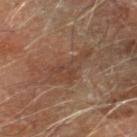Impression:
The lesion was photographed on a routine skin check and not biopsied; there is no pathology result.
Context:
Located on the right lower leg. A 15 mm close-up extracted from a 3D total-body photography capture. The tile uses cross-polarized illumination. The subject is a male aged 68–72.